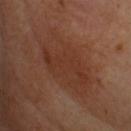<record>
  <biopsy_status>not biopsied; imaged during a skin examination</biopsy_status>
  <site>head or neck</site>
  <patient>
    <sex>female</sex>
    <age_approx>55</age_approx>
  </patient>
  <image>
    <source>total-body photography crop</source>
    <field_of_view_mm>15</field_of_view_mm>
  </image>
  <lesion_size>
    <long_diameter_mm_approx>8.0</long_diameter_mm_approx>
  </lesion_size>
  <lighting>cross-polarized</lighting>
</record>